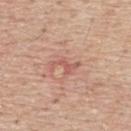Case summary:
• automated lesion analysis: a lesion color around L≈58 a*≈26 b*≈27 in CIELAB, a lesion–skin lightness drop of about 8, and a normalized lesion–skin contrast near 5.5; a border-irregularity rating of about 8/10
• acquisition: ~15 mm tile from a whole-body skin photo
• size: ~3.5 mm (longest diameter)
• body site: the upper back
• patient: male, roughly 55 years of age